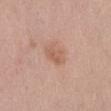| key | value |
|---|---|
| notes | imaged on a skin check; not biopsied |